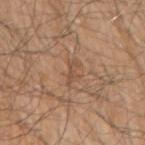- workup: no biopsy performed (imaged during a skin exam)
- tile lighting: white-light illumination
- lesion size: ~3 mm (longest diameter)
- anatomic site: the right upper arm
- imaging modality: ~15 mm tile from a whole-body skin photo
- TBP lesion metrics: a lesion–skin lightness drop of about 7 and a normalized lesion–skin contrast near 5.5; lesion-presence confidence of about 85/100
- subject: male, aged approximately 80